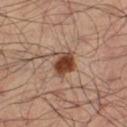{
  "biopsy_status": "not biopsied; imaged during a skin examination",
  "lighting": "cross-polarized",
  "site": "leg",
  "image": {
    "source": "total-body photography crop",
    "field_of_view_mm": 15
  },
  "automated_metrics": {
    "cielab_L": 42,
    "cielab_a": 21,
    "cielab_b": 29,
    "vs_skin_contrast_norm": 12.0,
    "color_variation_0_10": 4.5,
    "peripheral_color_asymmetry": 1.5
  },
  "lesion_size": {
    "long_diameter_mm_approx": 3.5
  },
  "patient": {
    "sex": "male",
    "age_approx": 50
  }
}